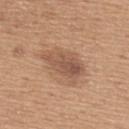| feature | finding |
|---|---|
| notes | catalogued during a skin exam; not biopsied |
| image source | 15 mm crop, total-body photography |
| anatomic site | the upper back |
| subject | male, aged approximately 65 |
| tile lighting | white-light illumination |